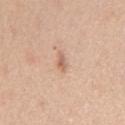subject = male, about 60 years old
illumination = white-light illumination
body site = the chest
size = ≈2.5 mm
automated metrics = a shape eccentricity near 0.9 and a symmetry-axis asymmetry near 0.3; an average lesion color of about L≈63 a*≈20 b*≈30 (CIELAB), a lesion–skin lightness drop of about 10, and a normalized lesion–skin contrast near 6.5; a border-irregularity rating of about 3/10, a color-variation rating of about 0/10, and peripheral color asymmetry of about 0; an automated nevus-likeness rating near 5 out of 100 and lesion-presence confidence of about 100/100
image = ~15 mm crop, total-body skin-cancer survey